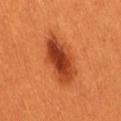Imaged during a routine full-body skin examination; the lesion was not biopsied and no histopathology is available. Approximately 6 mm at its widest. The total-body-photography lesion software estimated an automated nevus-likeness rating near 100 out of 100. The lesion is on the back. This image is a 15 mm lesion crop taken from a total-body photograph. The patient is a female approximately 30 years of age. Captured under cross-polarized illumination.